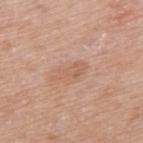Q: Is there a histopathology result?
A: total-body-photography surveillance lesion; no biopsy
Q: What is the imaging modality?
A: ~15 mm crop, total-body skin-cancer survey
Q: Automated lesion metrics?
A: a footprint of about 4.5 mm² and a shape eccentricity near 0.95; border irregularity of about 5 on a 0–10 scale, a color-variation rating of about 1/10, and a peripheral color-asymmetry measure near 0.5
Q: What is the anatomic site?
A: the upper back
Q: How was the tile lit?
A: white-light illumination
Q: Lesion size?
A: about 4 mm
Q: Patient demographics?
A: female, aged approximately 60